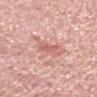Case summary:
- follow-up · catalogued during a skin exam; not biopsied